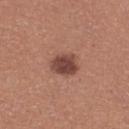A 15 mm crop from a total-body photograph taken for skin-cancer surveillance. The lesion is located on the right thigh. The patient is a female aged 38–42.